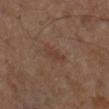Case summary:
– body site: the leg
– acquisition: total-body-photography crop, ~15 mm field of view
– patient: about 65 years old